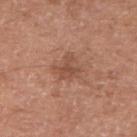Case summary:
• biopsy status — total-body-photography surveillance lesion; no biopsy
• image — ~15 mm crop, total-body skin-cancer survey
• lesion size — ~3 mm (longest diameter)
• TBP lesion metrics — a footprint of about 5.5 mm², a shape eccentricity near 0.5, and a symmetry-axis asymmetry near 0.55; a classifier nevus-likeness of about 0/100
• patient — male, in their mid- to late 60s
• body site — the arm